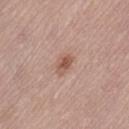Case summary:
– workup: total-body-photography surveillance lesion; no biopsy
– lesion size: ~3 mm (longest diameter)
– image-analysis metrics: a lesion area of about 4.5 mm², an eccentricity of roughly 0.8, and a shape-asymmetry score of about 0.2 (0 = symmetric); a border-irregularity rating of about 2/10 and peripheral color asymmetry of about 1; an automated nevus-likeness rating near 70 out of 100
– subject: female, aged 48–52
– image source: 15 mm crop, total-body photography
– lighting: white-light illumination
– anatomic site: the lower back